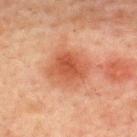Captured under cross-polarized illumination. The patient is a male about 60 years old. A roughly 15 mm field-of-view crop from a total-body skin photograph. From the upper back.